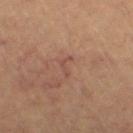This image is a 15 mm lesion crop taken from a total-body photograph.
Located on the right thigh.
Automated image analysis of the tile measured an average lesion color of about L≈46 a*≈21 b*≈25 (CIELAB), a lesion–skin lightness drop of about 6, and a lesion-to-skin contrast of about 5 (normalized; higher = more distinct).
A female patient aged around 65.
Captured under cross-polarized illumination.